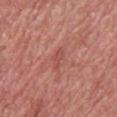Q: Was a biopsy performed?
A: total-body-photography surveillance lesion; no biopsy
Q: What is the imaging modality?
A: total-body-photography crop, ~15 mm field of view
Q: Patient demographics?
A: male, in their 60s
Q: What lighting was used for the tile?
A: white-light illumination
Q: What did automated image analysis measure?
A: lesion-presence confidence of about 95/100
Q: What is the lesion's diameter?
A: ~2.5 mm (longest diameter)
Q: Where on the body is the lesion?
A: the back